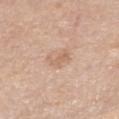Impression:
Part of a total-body skin-imaging series; this lesion was reviewed on a skin check and was not flagged for biopsy.
Acquisition and patient details:
The subject is a female aged approximately 55. On the right forearm. Imaged with white-light lighting. A 15 mm crop from a total-body photograph taken for skin-cancer surveillance. The lesion-visualizer software estimated an area of roughly 4 mm², an outline eccentricity of about 0.6 (0 = round, 1 = elongated), and two-axis asymmetry of about 0.45. And it measured a lesion color around L≈63 a*≈18 b*≈31 in CIELAB, about 7 CIELAB-L* units darker than the surrounding skin, and a normalized border contrast of about 5. It also reported a border-irregularity rating of about 6/10, a within-lesion color-variation index near 2/10, and radial color variation of about 0.5. The software also gave a nevus-likeness score of about 0/100. The recorded lesion diameter is about 3 mm.